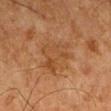Impression: No biopsy was performed on this lesion — it was imaged during a full skin examination and was not determined to be concerning. Acquisition and patient details: A 15 mm close-up tile from a total-body photography series done for melanoma screening. Imaged with cross-polarized lighting. Located on the right thigh. A male patient, roughly 80 years of age. Measured at roughly 6 mm in maximum diameter.